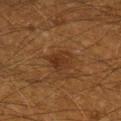{
  "biopsy_status": "not biopsied; imaged during a skin examination",
  "image": {
    "source": "total-body photography crop",
    "field_of_view_mm": 15
  },
  "lighting": "cross-polarized",
  "patient": {
    "sex": "male",
    "age_approx": 60
  },
  "automated_metrics": {
    "area_mm2_approx": 5.5,
    "eccentricity": 0.65,
    "shape_asymmetry": 0.3,
    "cielab_L": 27,
    "cielab_a": 17,
    "cielab_b": 27,
    "vs_skin_darker_L": 6.0,
    "vs_skin_contrast_norm": 6.5
  },
  "site": "right lower leg"
}